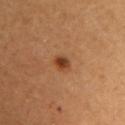workup: no biopsy performed (imaged during a skin exam)
lighting: cross-polarized illumination
acquisition: 15 mm crop, total-body photography
site: the left upper arm
patient: female, approximately 50 years of age